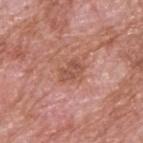The lesion was tiled from a total-body skin photograph and was not biopsied. The tile uses white-light illumination. The lesion is located on the upper back. The subject is a male roughly 60 years of age. A roughly 15 mm field-of-view crop from a total-body skin photograph.